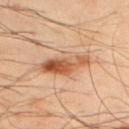Recorded during total-body skin imaging; not selected for excision or biopsy.
Cropped from a whole-body photographic skin survey; the tile spans about 15 mm.
The total-body-photography lesion software estimated a lesion area of about 10 mm², an eccentricity of roughly 0.95, and two-axis asymmetry of about 0.3. It also reported a border-irregularity rating of about 3.5/10, a within-lesion color-variation index near 10/10, and peripheral color asymmetry of about 4. The analysis additionally found a detector confidence of about 100 out of 100 that the crop contains a lesion.
The lesion's longest dimension is about 6 mm.
A male subject, roughly 50 years of age.
On the upper back.
This is a cross-polarized tile.